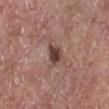<record>
<biopsy_status>not biopsied; imaged during a skin examination</biopsy_status>
<site>right lower leg</site>
<image>
  <source>total-body photography crop</source>
  <field_of_view_mm>15</field_of_view_mm>
</image>
<lighting>white-light</lighting>
<patient>
  <sex>male</sex>
  <age_approx>75</age_approx>
</patient>
<lesion_size>
  <long_diameter_mm_approx>2.5</long_diameter_mm_approx>
</lesion_size>
</record>